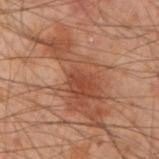Clinical impression: This lesion was catalogued during total-body skin photography and was not selected for biopsy. Context: The lesion is on the left arm. A male patient about 50 years old. This image is a 15 mm lesion crop taken from a total-body photograph.